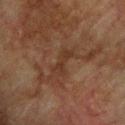biopsy status: no biopsy performed (imaged during a skin exam).